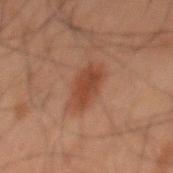Case summary:
* notes: catalogued during a skin exam; not biopsied
* patient: male, aged around 60
* imaging modality: total-body-photography crop, ~15 mm field of view
* lighting: cross-polarized illumination
* size: ≈4 mm
* anatomic site: the back